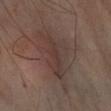{
  "site": "arm",
  "lesion_size": {
    "long_diameter_mm_approx": 8.5
  },
  "image": {
    "source": "total-body photography crop",
    "field_of_view_mm": 15
  }
}